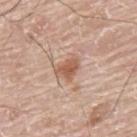| feature | finding |
|---|---|
| biopsy status | imaged on a skin check; not biopsied |
| subject | male, approximately 75 years of age |
| image source | 15 mm crop, total-body photography |
| location | the upper back |
| lighting | white-light illumination |
| lesion diameter | ~3 mm (longest diameter) |